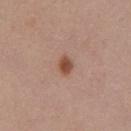Q: Was a biopsy performed?
A: no biopsy performed (imaged during a skin exam)
Q: What is the lesion's diameter?
A: about 2.5 mm
Q: Lesion location?
A: the chest
Q: How was this image acquired?
A: ~15 mm tile from a whole-body skin photo
Q: What did automated image analysis measure?
A: a footprint of about 3.5 mm² and an eccentricity of roughly 0.75; an average lesion color of about L≈50 a*≈22 b*≈29 (CIELAB), about 12 CIELAB-L* units darker than the surrounding skin, and a normalized border contrast of about 9; an automated nevus-likeness rating near 95 out of 100
Q: Who is the patient?
A: male, approximately 55 years of age